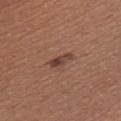Clinical impression: Recorded during total-body skin imaging; not selected for excision or biopsy. Image and clinical context: Captured under white-light illumination. A lesion tile, about 15 mm wide, cut from a 3D total-body photograph. The lesion's longest dimension is about 3 mm. The patient is a male aged around 45. Located on the chest.